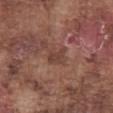| key | value |
|---|---|
| biopsy status | no biopsy performed (imaged during a skin exam) |
| anatomic site | the abdomen |
| automated metrics | a border-irregularity index near 3/10 and a color-variation rating of about 1/10; a lesion-detection confidence of about 100/100 |
| illumination | white-light illumination |
| lesion diameter | ~2.5 mm (longest diameter) |
| image | ~15 mm tile from a whole-body skin photo |
| subject | male, aged around 75 |